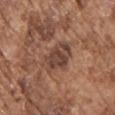biopsy_status: not biopsied; imaged during a skin examination
site: chest
image:
  source: total-body photography crop
  field_of_view_mm: 15
patient:
  sex: male
  age_approx: 75
lesion_size:
  long_diameter_mm_approx: 4.5
automated_metrics:
  vs_skin_darker_L: 10.0
  vs_skin_contrast_norm: 8.5
  nevus_likeness_0_100: 25
lighting: white-light